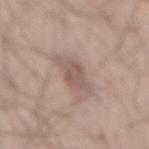Recorded during total-body skin imaging; not selected for excision or biopsy.
From the mid back.
Imaged with white-light lighting.
A region of skin cropped from a whole-body photographic capture, roughly 15 mm wide.
The recorded lesion diameter is about 4 mm.
The patient is a male aged around 55.
The lesion-visualizer software estimated a footprint of about 7.5 mm², an outline eccentricity of about 0.85 (0 = round, 1 = elongated), and a symmetry-axis asymmetry near 0.3. And it measured internal color variation of about 3 on a 0–10 scale and a peripheral color-asymmetry measure near 1. The analysis additionally found a classifier nevus-likeness of about 0/100 and a lesion-detection confidence of about 90/100.